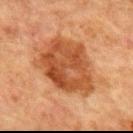biopsy status = catalogued during a skin exam; not biopsied
image-analysis metrics = internal color variation of about 5.5 on a 0–10 scale and peripheral color asymmetry of about 2; a classifier nevus-likeness of about 80/100 and a lesion-detection confidence of about 100/100
patient = male, aged 63 to 67
image = 15 mm crop, total-body photography
tile lighting = cross-polarized
body site = the chest
size = ~8 mm (longest diameter)